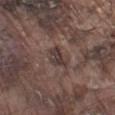Clinical summary:
A close-up tile cropped from a whole-body skin photograph, about 15 mm across. The recorded lesion diameter is about 3 mm. The lesion is located on the left lower leg. Captured under white-light illumination. A male patient aged approximately 75.